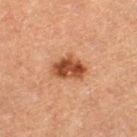Captured during whole-body skin photography for melanoma surveillance; the lesion was not biopsied.
A 15 mm crop from a total-body photograph taken for skin-cancer surveillance.
The lesion is located on the left thigh.
The subject is a female approximately 60 years of age.